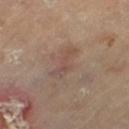This image is a 15 mm lesion crop taken from a total-body photograph.
The subject is a male aged around 65.
Approximately 4.5 mm at its widest.
Automated tile analysis of the lesion measured an area of roughly 6.5 mm² and two-axis asymmetry of about 0.4. It also reported roughly 6 lightness units darker than nearby skin and a normalized lesion–skin contrast near 5. The software also gave an automated nevus-likeness rating near 0 out of 100 and a detector confidence of about 100 out of 100 that the crop contains a lesion.
On the left thigh.